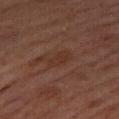Case summary:
- notes: total-body-photography surveillance lesion; no biopsy
- subject: male, about 60 years old
- lesion diameter: ~3 mm (longest diameter)
- anatomic site: the right thigh
- image: ~15 mm tile from a whole-body skin photo
- lighting: cross-polarized illumination
- image-analysis metrics: roughly 4 lightness units darker than nearby skin and a normalized border contrast of about 5.5; a border-irregularity rating of about 3/10, internal color variation of about 1.5 on a 0–10 scale, and a peripheral color-asymmetry measure near 0.5